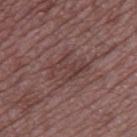Notes:
• notes — catalogued during a skin exam; not biopsied
• subject — male, aged around 50
• location — the right thigh
• image — 15 mm crop, total-body photography